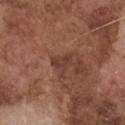Imaged during a routine full-body skin examination; the lesion was not biopsied and no histopathology is available.
About 2.5 mm across.
A male subject roughly 75 years of age.
The total-body-photography lesion software estimated a lesion area of about 4 mm², an outline eccentricity of about 0.75 (0 = round, 1 = elongated), and two-axis asymmetry of about 0.3. The analysis additionally found roughly 8 lightness units darker than nearby skin and a normalized border contrast of about 7. The analysis additionally found a border-irregularity index near 2.5/10 and a within-lesion color-variation index near 2/10.
Imaged with white-light lighting.
A region of skin cropped from a whole-body photographic capture, roughly 15 mm wide.
On the front of the torso.